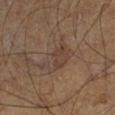Assessment:
Imaged during a routine full-body skin examination; the lesion was not biopsied and no histopathology is available.